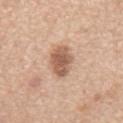A 15 mm crop from a total-body photograph taken for skin-cancer surveillance. From the abdomen. A male patient aged 63–67. The recorded lesion diameter is about 4.5 mm. Imaged with white-light lighting. The lesion-visualizer software estimated a lesion color around L≈58 a*≈20 b*≈30 in CIELAB, a lesion–skin lightness drop of about 14, and a normalized lesion–skin contrast near 8.5. The analysis additionally found a border-irregularity rating of about 2/10 and radial color variation of about 1.